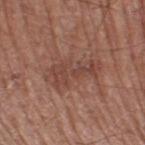The lesion was tiled from a total-body skin photograph and was not biopsied.
A male subject, approximately 70 years of age.
About 6 mm across.
A 15 mm close-up tile from a total-body photography series done for melanoma screening.
Located on the leg.
Imaged with white-light lighting.